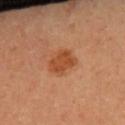Assessment: Recorded during total-body skin imaging; not selected for excision or biopsy. Context: Captured under cross-polarized illumination. A roughly 15 mm field-of-view crop from a total-body skin photograph. A female patient in their 50s.